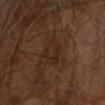Impression:
The lesion was photographed on a routine skin check and not biopsied; there is no pathology result.
Context:
The lesion is on the left arm. A male subject about 60 years old. This image is a 15 mm lesion crop taken from a total-body photograph.